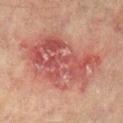| feature | finding |
|---|---|
| image source | 15 mm crop, total-body photography |
| tile lighting | cross-polarized illumination |
| automated metrics | a lesion area of about 44 mm², a shape eccentricity near 0.85, and a symmetry-axis asymmetry near 0.3; a border-irregularity rating of about 4.5/10, a within-lesion color-variation index near 8/10, and a peripheral color-asymmetry measure near 3; an automated nevus-likeness rating near 0 out of 100 and a detector confidence of about 100 out of 100 that the crop contains a lesion |
| site | the right lower leg |
| patient | male, in their mid-70s |
| lesion diameter | ≈11 mm |